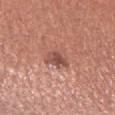The lesion was tiled from a total-body skin photograph and was not biopsied.
The recorded lesion diameter is about 3.5 mm.
The lesion-visualizer software estimated a lesion area of about 8 mm² and an eccentricity of roughly 0.7. And it measured internal color variation of about 6 on a 0–10 scale and peripheral color asymmetry of about 2.
The subject is a male aged 43–47.
A lesion tile, about 15 mm wide, cut from a 3D total-body photograph.
The tile uses white-light illumination.
On the left upper arm.